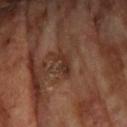No biopsy was performed on this lesion — it was imaged during a full skin examination and was not determined to be concerning. A 15 mm close-up extracted from a 3D total-body photography capture. From the upper back. A male patient, aged 68–72. The tile uses cross-polarized illumination. Measured at roughly 3 mm in maximum diameter.